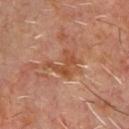Notes:
* biopsy status — imaged on a skin check; not biopsied
* patient — male, roughly 60 years of age
* location — the chest
* diameter — ≈4.5 mm
* tile lighting — cross-polarized illumination
* acquisition — ~15 mm crop, total-body skin-cancer survey